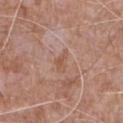Findings:
• follow-up: catalogued during a skin exam; not biopsied
• location: the upper back
• subject: male, aged approximately 60
• lighting: white-light illumination
• acquisition: total-body-photography crop, ~15 mm field of view
• lesion diameter: about 3 mm